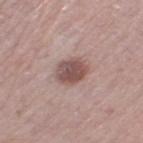Assessment:
The lesion was tiled from a total-body skin photograph and was not biopsied.
Image and clinical context:
Automated image analysis of the tile measured border irregularity of about 1.5 on a 0–10 scale. A female subject, roughly 65 years of age. A 15 mm close-up tile from a total-body photography series done for melanoma screening. This is a white-light tile. The recorded lesion diameter is about 4 mm. The lesion is located on the left thigh.